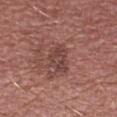{
  "biopsy_status": "not biopsied; imaged during a skin examination",
  "patient": {
    "sex": "male",
    "age_approx": 55
  },
  "image": {
    "source": "total-body photography crop",
    "field_of_view_mm": 15
  },
  "site": "right upper arm"
}